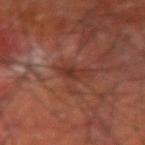Q: Was a biopsy performed?
A: catalogued during a skin exam; not biopsied
Q: What is the anatomic site?
A: the right upper arm
Q: Illumination type?
A: cross-polarized illumination
Q: Who is the patient?
A: male, roughly 60 years of age
Q: What did automated image analysis measure?
A: a normalized lesion–skin contrast near 6.5; a nevus-likeness score of about 0/100
Q: Lesion size?
A: ≈3 mm
Q: What kind of image is this?
A: 15 mm crop, total-body photography